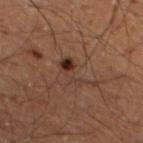The lesion was photographed on a routine skin check and not biopsied; there is no pathology result. A male subject, approximately 50 years of age. Located on the left lower leg. A close-up tile cropped from a whole-body skin photograph, about 15 mm across.